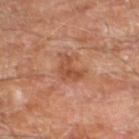{"biopsy_status": "not biopsied; imaged during a skin examination", "image": {"source": "total-body photography crop", "field_of_view_mm": 15}, "site": "left lower leg", "patient": {"sex": "male", "age_approx": 70}, "lighting": "cross-polarized", "automated_metrics": {"area_mm2_approx": 5.5, "eccentricity": 0.8, "shape_asymmetry": 0.5, "vs_skin_darker_L": 9.0, "vs_skin_contrast_norm": 6.5, "border_irregularity_0_10": 5.5, "color_variation_0_10": 3.5, "peripheral_color_asymmetry": 1.5, "lesion_detection_confidence_0_100": 100}}